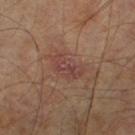This lesion was catalogued during total-body skin photography and was not selected for biopsy. The lesion is located on the right leg. The recorded lesion diameter is about 4.5 mm. A male patient, approximately 60 years of age. A roughly 15 mm field-of-view crop from a total-body skin photograph. The tile uses cross-polarized illumination.